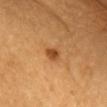Impression: The lesion was tiled from a total-body skin photograph and was not biopsied. Context: A lesion tile, about 15 mm wide, cut from a 3D total-body photograph. A female patient, aged 63 to 67. The lesion is on the chest. This is a cross-polarized tile. The total-body-photography lesion software estimated an average lesion color of about L≈47 a*≈25 b*≈42 (CIELAB). And it measured a border-irregularity rating of about 2/10 and internal color variation of about 2 on a 0–10 scale.